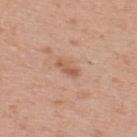workup=imaged on a skin check; not biopsied | site=the back | acquisition=15 mm crop, total-body photography | lesion diameter=≈3 mm | image-analysis metrics=a footprint of about 2.5 mm², a shape eccentricity near 0.95, and two-axis asymmetry of about 0.3; a mean CIELAB color near L≈57 a*≈22 b*≈32 and roughly 9 lightness units darker than nearby skin; a within-lesion color-variation index near 0/10 and peripheral color asymmetry of about 0 | subject=male, about 30 years old | illumination=white-light.